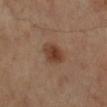Q: Is there a histopathology result?
A: no biopsy performed (imaged during a skin exam)
Q: Where on the body is the lesion?
A: the left leg
Q: What kind of image is this?
A: ~15 mm tile from a whole-body skin photo
Q: Who is the patient?
A: male, in their 50s